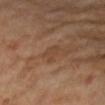{"biopsy_status": "not biopsied; imaged during a skin examination", "automated_metrics": {"lesion_detection_confidence_0_100": 100}, "patient": {"sex": "female", "age_approx": 65}, "site": "arm", "image": {"source": "total-body photography crop", "field_of_view_mm": 15}}